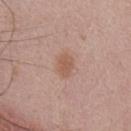notes: catalogued during a skin exam; not biopsied
lighting: white-light
patient: male, aged around 25
body site: the chest
imaging modality: total-body-photography crop, ~15 mm field of view